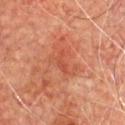Notes:
• anatomic site · the front of the torso
• TBP lesion metrics · an area of roughly 2.5 mm²; about 6 CIELAB-L* units darker than the surrounding skin and a lesion-to-skin contrast of about 5 (normalized; higher = more distinct)
• patient · male, aged 73 to 77
• imaging modality · 15 mm crop, total-body photography